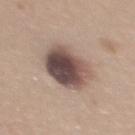| field | value |
|---|---|
| biopsy status | no biopsy performed (imaged during a skin exam) |
| acquisition | ~15 mm tile from a whole-body skin photo |
| patient | female, aged around 35 |
| body site | the back |
| lighting | white-light |
| lesion size | about 6 mm |